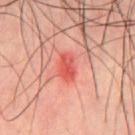Image and clinical context: A roughly 15 mm field-of-view crop from a total-body skin photograph. The lesion is located on the chest. This is a cross-polarized tile. A male patient about 45 years old.